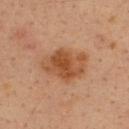Impression: The lesion was tiled from a total-body skin photograph and was not biopsied. Context: A male subject aged 33 to 37. Located on the upper back. Cropped from a whole-body photographic skin survey; the tile spans about 15 mm.